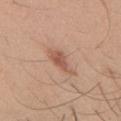This lesion was catalogued during total-body skin photography and was not selected for biopsy. The recorded lesion diameter is about 4 mm. The lesion is on the chest. Cropped from a whole-body photographic skin survey; the tile spans about 15 mm. A male patient, approximately 30 years of age.